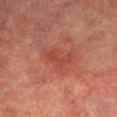Case summary:
• notes · total-body-photography surveillance lesion; no biopsy
• lighting · cross-polarized
• location · the left lower leg
• subject · male, about 75 years old
• acquisition · ~15 mm crop, total-body skin-cancer survey